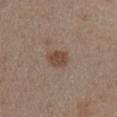follow-up: imaged on a skin check; not biopsied | acquisition: ~15 mm crop, total-body skin-cancer survey | anatomic site: the right upper arm | subject: male, approximately 55 years of age.